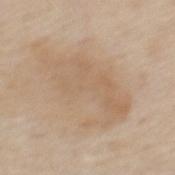Assessment:
This lesion was catalogued during total-body skin photography and was not selected for biopsy.
Context:
A 15 mm close-up tile from a total-body photography series done for melanoma screening. Automated image analysis of the tile measured roughly 6 lightness units darker than nearby skin and a normalized lesion–skin contrast near 5. The software also gave internal color variation of about 3 on a 0–10 scale and a peripheral color-asymmetry measure near 1. Imaged with white-light lighting. About 9 mm across. The subject is a male approximately 65 years of age. The lesion is on the mid back.